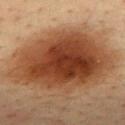body site: the mid back | automated metrics: a lesion color around L≈39 a*≈23 b*≈32 in CIELAB, roughly 15 lightness units darker than nearby skin, and a lesion-to-skin contrast of about 12 (normalized; higher = more distinct); a border-irregularity index near 2.5/10, a color-variation rating of about 7/10, and radial color variation of about 2; an automated nevus-likeness rating near 100 out of 100 and a detector confidence of about 100 out of 100 that the crop contains a lesion | illumination: cross-polarized | patient: male, aged around 35 | lesion diameter: about 12 mm | image: ~15 mm crop, total-body skin-cancer survey.